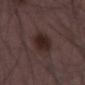workup = no biopsy performed (imaged during a skin exam) | subject = male, roughly 50 years of age | lighting = white-light illumination | imaging modality = ~15 mm tile from a whole-body skin photo | body site = the left thigh | automated lesion analysis = a lesion color around L≈25 a*≈15 b*≈16 in CIELAB and roughly 8 lightness units darker than nearby skin.